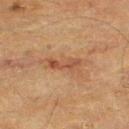A male patient, aged 83 or over.
The total-body-photography lesion software estimated a footprint of about 5 mm². It also reported a mean CIELAB color near L≈44 a*≈21 b*≈30 and a lesion–skin lightness drop of about 8. The analysis additionally found a border-irregularity rating of about 4.5/10, internal color variation of about 2.5 on a 0–10 scale, and radial color variation of about 0.5. It also reported lesion-presence confidence of about 100/100.
Cropped from a whole-body photographic skin survey; the tile spans about 15 mm.
From the left thigh.